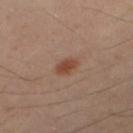{"biopsy_status": "not biopsied; imaged during a skin examination", "site": "leg", "image": {"source": "total-body photography crop", "field_of_view_mm": 15}, "patient": {"sex": "female", "age_approx": 40}}